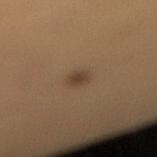Clinical impression: The lesion was tiled from a total-body skin photograph and was not biopsied. Image and clinical context: Automated tile analysis of the lesion measured an area of roughly 3.5 mm² and an eccentricity of roughly 0.6. The analysis additionally found a classifier nevus-likeness of about 95/100 and a lesion-detection confidence of about 100/100. On the right lower leg. The patient is a female aged approximately 25. A lesion tile, about 15 mm wide, cut from a 3D total-body photograph. Imaged with cross-polarized lighting.